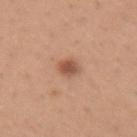<record>
  <biopsy_status>not biopsied; imaged during a skin examination</biopsy_status>
  <lighting>white-light</lighting>
  <patient>
    <sex>female</sex>
    <age_approx>30</age_approx>
  </patient>
  <automated_metrics>
    <area_mm2_approx>4.0</area_mm2_approx>
    <eccentricity>0.7</eccentricity>
    <shape_asymmetry>0.2</shape_asymmetry>
    <cielab_L>53</cielab_L>
    <cielab_a>23</cielab_a>
    <cielab_b>31</cielab_b>
    <vs_skin_darker_L>12.0</vs_skin_darker_L>
    <nevus_likeness_0_100>90</nevus_likeness_0_100>
    <lesion_detection_confidence_0_100>100</lesion_detection_confidence_0_100>
  </automated_metrics>
  <site>right upper arm</site>
  <image>
    <source>total-body photography crop</source>
    <field_of_view_mm>15</field_of_view_mm>
  </image>
</record>